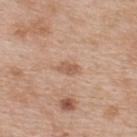Case summary:
• patient: female, about 40 years old
• imaging modality: total-body-photography crop, ~15 mm field of view
• lesion size: ≈2.5 mm
• automated metrics: an area of roughly 3 mm², a shape eccentricity near 0.85, and two-axis asymmetry of about 0.35; a mean CIELAB color near L≈58 a*≈20 b*≈31, a lesion–skin lightness drop of about 9, and a lesion-to-skin contrast of about 6.5 (normalized; higher = more distinct); a border-irregularity index near 3.5/10 and internal color variation of about 2 on a 0–10 scale
• site: the upper back
• lighting: white-light